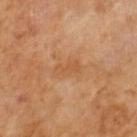Image and clinical context: A male subject, approximately 65 years of age. This image is a 15 mm lesion crop taken from a total-body photograph.